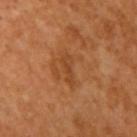The lesion was tiled from a total-body skin photograph and was not biopsied.
Measured at roughly 3.5 mm in maximum diameter.
Imaged with cross-polarized lighting.
A roughly 15 mm field-of-view crop from a total-body skin photograph.
A female patient, approximately 55 years of age.
From the right upper arm.